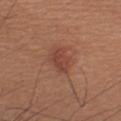Part of a total-body skin-imaging series; this lesion was reviewed on a skin check and was not flagged for biopsy. A male subject, aged 53 to 57. The recorded lesion diameter is about 3.5 mm. A 15 mm crop from a total-body photograph taken for skin-cancer surveillance. The lesion is located on the left upper arm. The tile uses white-light illumination.